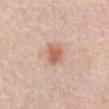| key | value |
|---|---|
| notes | catalogued during a skin exam; not biopsied |
| lighting | white-light illumination |
| imaging modality | ~15 mm tile from a whole-body skin photo |
| subject | male, aged 73 to 77 |
| lesion size | about 2.5 mm |
| body site | the abdomen |